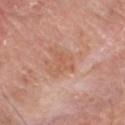Q: Was this lesion biopsied?
A: imaged on a skin check; not biopsied
Q: How was this image acquired?
A: ~15 mm crop, total-body skin-cancer survey
Q: What did automated image analysis measure?
A: a mean CIELAB color near L≈58 a*≈24 b*≈32 and a normalized border contrast of about 5
Q: Lesion size?
A: ~4 mm (longest diameter)
Q: What are the patient's age and sex?
A: male, aged approximately 80
Q: What is the anatomic site?
A: the head or neck